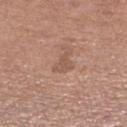Imaged during a routine full-body skin examination; the lesion was not biopsied and no histopathology is available.
The total-body-photography lesion software estimated a lesion area of about 4 mm², an outline eccentricity of about 0.75 (0 = round, 1 = elongated), and a symmetry-axis asymmetry near 0.5. And it measured a mean CIELAB color near L≈54 a*≈19 b*≈29, roughly 7 lightness units darker than nearby skin, and a normalized lesion–skin contrast near 5. The analysis additionally found a border-irregularity rating of about 5/10 and internal color variation of about 1 on a 0–10 scale. It also reported a lesion-detection confidence of about 100/100.
Longest diameter approximately 3 mm.
A male subject in their mid- to late 70s.
On the right lower leg.
A 15 mm close-up extracted from a 3D total-body photography capture.
Imaged with white-light lighting.